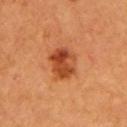Notes:
- notes — imaged on a skin check; not biopsied
- image — 15 mm crop, total-body photography
- subject — female, approximately 60 years of age
- body site — the left upper arm